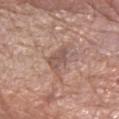Imaged during a routine full-body skin examination; the lesion was not biopsied and no histopathology is available. A female subject roughly 55 years of age. Cropped from a total-body skin-imaging series; the visible field is about 15 mm. On the left forearm.